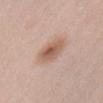The lesion was tiled from a total-body skin photograph and was not biopsied. A region of skin cropped from a whole-body photographic capture, roughly 15 mm wide. A female patient, aged approximately 40. The lesion's longest dimension is about 4.5 mm. The tile uses white-light illumination. The total-body-photography lesion software estimated a lesion area of about 9 mm². It also reported a border-irregularity rating of about 2.5/10 and a within-lesion color-variation index near 5/10. The software also gave a nevus-likeness score of about 90/100 and a lesion-detection confidence of about 100/100. On the abdomen.